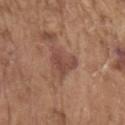Impression: Imaged during a routine full-body skin examination; the lesion was not biopsied and no histopathology is available. Acquisition and patient details: Imaged with white-light lighting. A male subject aged around 80. A roughly 15 mm field-of-view crop from a total-body skin photograph. The total-body-photography lesion software estimated a border-irregularity rating of about 4.5/10 and a color-variation rating of about 2.5/10. Measured at roughly 3.5 mm in maximum diameter. On the left upper arm.